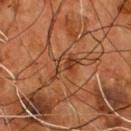Assessment: Part of a total-body skin-imaging series; this lesion was reviewed on a skin check and was not flagged for biopsy. Acquisition and patient details: The recorded lesion diameter is about 4 mm. The tile uses cross-polarized illumination. From the chest. A roughly 15 mm field-of-view crop from a total-body skin photograph. The lesion-visualizer software estimated an area of roughly 5.5 mm², a shape eccentricity near 0.85, and a shape-asymmetry score of about 0.5 (0 = symmetric). And it measured a lesion–skin lightness drop of about 8 and a normalized lesion–skin contrast near 6.5. The software also gave border irregularity of about 5.5 on a 0–10 scale, a within-lesion color-variation index near 5/10, and a peripheral color-asymmetry measure near 1.5. And it measured a classifier nevus-likeness of about 15/100 and a lesion-detection confidence of about 90/100. A male subject aged 53 to 57.